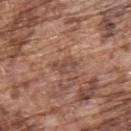Clinical summary: This is a white-light tile. The lesion's longest dimension is about 3 mm. Cropped from a whole-body photographic skin survey; the tile spans about 15 mm. An algorithmic analysis of the crop reported a footprint of about 4.5 mm² and two-axis asymmetry of about 0.3. And it measured roughly 7 lightness units darker than nearby skin and a lesion-to-skin contrast of about 6 (normalized; higher = more distinct). It also reported a border-irregularity rating of about 3.5/10 and peripheral color asymmetry of about 1. It also reported a classifier nevus-likeness of about 0/100 and a lesion-detection confidence of about 80/100. A male subject, aged 73 to 77. The lesion is located on the upper back.